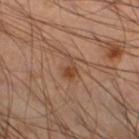Captured during whole-body skin photography for melanoma surveillance; the lesion was not biopsied.
About 3 mm across.
On the leg.
A 15 mm crop from a total-body photograph taken for skin-cancer surveillance.
A male patient, roughly 50 years of age.
Imaged with cross-polarized lighting.